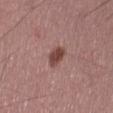biopsy status: catalogued during a skin exam; not biopsied | lesion diameter: ≈3 mm | imaging modality: ~15 mm tile from a whole-body skin photo | subject: male, about 55 years old | illumination: white-light illumination | site: the right thigh.